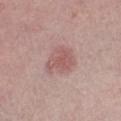{
  "biopsy_status": "not biopsied; imaged during a skin examination",
  "patient": {
    "sex": "female",
    "age_approx": 65
  },
  "image": {
    "source": "total-body photography crop",
    "field_of_view_mm": 15
  },
  "lighting": "white-light",
  "automated_metrics": {
    "area_mm2_approx": 9.0,
    "eccentricity": 0.55,
    "cielab_L": 56,
    "cielab_a": 22,
    "cielab_b": 21,
    "vs_skin_contrast_norm": 6.0,
    "border_irregularity_0_10": 3.5,
    "color_variation_0_10": 2.0,
    "peripheral_color_asymmetry": 0.5
  },
  "site": "leg"
}